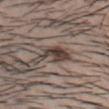| key | value |
|---|---|
| anatomic site | the chest |
| subject | male, in their mid-30s |
| imaging modality | 15 mm crop, total-body photography |
| lighting | white-light |